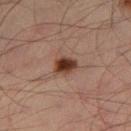Captured during whole-body skin photography for melanoma surveillance; the lesion was not biopsied.
The lesion is on the left thigh.
Captured under cross-polarized illumination.
A male patient aged approximately 35.
Cropped from a whole-body photographic skin survey; the tile spans about 15 mm.
Approximately 3 mm at its widest.